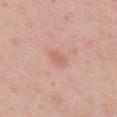Case summary:
• workup: no biopsy performed (imaged during a skin exam)
• image source: 15 mm crop, total-body photography
• illumination: white-light
• location: the upper back
• subject: female, approximately 50 years of age